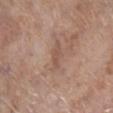Recorded during total-body skin imaging; not selected for excision or biopsy. Automated image analysis of the tile measured a lesion color around L≈52 a*≈18 b*≈27 in CIELAB, roughly 6 lightness units darker than nearby skin, and a normalized border contrast of about 5. A female patient in their mid-80s or older. Located on the right lower leg. Captured under white-light illumination. The recorded lesion diameter is about 2.5 mm. A 15 mm crop from a total-body photograph taken for skin-cancer surveillance.